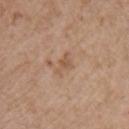Findings:
* notes — catalogued during a skin exam; not biopsied
* patient — male, in their mid- to late 60s
* body site — the right upper arm
* image — 15 mm crop, total-body photography